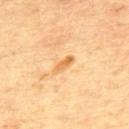Impression: This lesion was catalogued during total-body skin photography and was not selected for biopsy. Acquisition and patient details: The subject is a male aged around 65. This image is a 15 mm lesion crop taken from a total-body photograph. Located on the back.